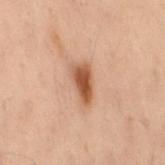Image and clinical context: From the back. A lesion tile, about 15 mm wide, cut from a 3D total-body photograph. The subject is a female in their mid- to late 50s.